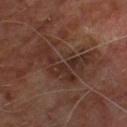Impression:
Imaged during a routine full-body skin examination; the lesion was not biopsied and no histopathology is available.
Image and clinical context:
A roughly 15 mm field-of-view crop from a total-body skin photograph. Captured under cross-polarized illumination. On the back. Measured at roughly 8 mm in maximum diameter. The subject is a male roughly 60 years of age.